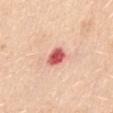biopsy status: no biopsy performed (imaged during a skin exam)
TBP lesion metrics: an average lesion color of about L≈60 a*≈36 b*≈28 (CIELAB), a lesion–skin lightness drop of about 19, and a normalized lesion–skin contrast near 11
image source: ~15 mm tile from a whole-body skin photo
site: the mid back
illumination: white-light
patient: female, aged approximately 55